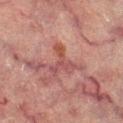| key | value |
|---|---|
| biopsy status | no biopsy performed (imaged during a skin exam) |
| patient | female, in their 70s |
| imaging modality | ~15 mm crop, total-body skin-cancer survey |
| location | the leg |